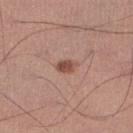Background:
From the leg. About 2.5 mm across. A 15 mm crop from a total-body photograph taken for skin-cancer surveillance. The tile uses white-light illumination. A male subject, about 55 years old. Automated image analysis of the tile measured an eccentricity of roughly 0.8 and two-axis asymmetry of about 0.25. And it measured border irregularity of about 2 on a 0–10 scale, a color-variation rating of about 2.5/10, and a peripheral color-asymmetry measure near 1. And it measured an automated nevus-likeness rating near 95 out of 100 and a detector confidence of about 100 out of 100 that the crop contains a lesion.